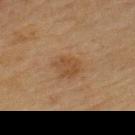No biopsy was performed on this lesion — it was imaged during a full skin examination and was not determined to be concerning. A male patient roughly 70 years of age. The total-body-photography lesion software estimated a lesion color around L≈38 a*≈16 b*≈29 in CIELAB and a lesion-to-skin contrast of about 6 (normalized; higher = more distinct). And it measured a lesion-detection confidence of about 100/100. A close-up tile cropped from a whole-body skin photograph, about 15 mm across. Captured under cross-polarized illumination. From the upper back. About 3.5 mm across.